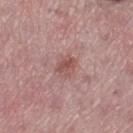* notes · total-body-photography surveillance lesion; no biopsy
* image source · ~15 mm tile from a whole-body skin photo
* location · the left thigh
* patient · female, aged 43–47